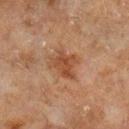Case summary:
– biopsy status · total-body-photography surveillance lesion; no biopsy
– anatomic site · the left lower leg
– subject · male, aged 63 to 67
– lighting · cross-polarized
– imaging modality · total-body-photography crop, ~15 mm field of view
– diameter · about 3.5 mm